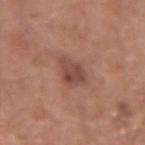Part of a total-body skin-imaging series; this lesion was reviewed on a skin check and was not flagged for biopsy.
A close-up tile cropped from a whole-body skin photograph, about 15 mm across.
An algorithmic analysis of the crop reported a footprint of about 6 mm² and an outline eccentricity of about 0.45 (0 = round, 1 = elongated). It also reported an average lesion color of about L≈46 a*≈22 b*≈25 (CIELAB), a lesion–skin lightness drop of about 10, and a lesion-to-skin contrast of about 7.5 (normalized; higher = more distinct). The software also gave a border-irregularity rating of about 3/10, internal color variation of about 4 on a 0–10 scale, and a peripheral color-asymmetry measure near 1.5. And it measured a classifier nevus-likeness of about 10/100 and a detector confidence of about 100 out of 100 that the crop contains a lesion.
Approximately 3 mm at its widest.
A male subject approximately 55 years of age.
The lesion is on the left forearm.